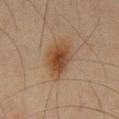Captured during whole-body skin photography for melanoma surveillance; the lesion was not biopsied.
On the chest.
Automated tile analysis of the lesion measured a border-irregularity rating of about 2.5/10, internal color variation of about 5 on a 0–10 scale, and a peripheral color-asymmetry measure near 1.5. The software also gave an automated nevus-likeness rating near 100 out of 100 and lesion-presence confidence of about 100/100.
A lesion tile, about 15 mm wide, cut from a 3D total-body photograph.
A male subject in their mid-60s.
The recorded lesion diameter is about 5.5 mm.